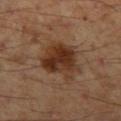<lesion>
  <biopsy_status>not biopsied; imaged during a skin examination</biopsy_status>
  <lesion_size>
    <long_diameter_mm_approx>5.5</long_diameter_mm_approx>
  </lesion_size>
  <patient>
    <sex>male</sex>
    <age_approx>55</age_approx>
  </patient>
  <image>
    <source>total-body photography crop</source>
    <field_of_view_mm>15</field_of_view_mm>
  </image>
  <site>left thigh</site>
  <lighting>cross-polarized</lighting>
  <automated_metrics>
    <eccentricity>0.55</eccentricity>
    <shape_asymmetry>0.3</shape_asymmetry>
    <border_irregularity_0_10>3.5</border_irregularity_0_10>
    <color_variation_0_10>6.0</color_variation_0_10>
    <peripheral_color_asymmetry>2.0</peripheral_color_asymmetry>
    <nevus_likeness_0_100>90</nevus_likeness_0_100>
  </automated_metrics>
</lesion>